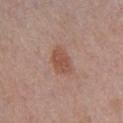image source: ~15 mm tile from a whole-body skin photo | subject: male, aged 38–42 | lesion size: about 3.5 mm | anatomic site: the chest | lighting: white-light.